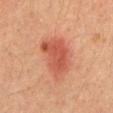follow-up: catalogued during a skin exam; not biopsied | site: the back | image source: 15 mm crop, total-body photography | subject: male, in their mid-60s.